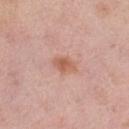This lesion was catalogued during total-body skin photography and was not selected for biopsy. The lesion is located on the left thigh. A close-up tile cropped from a whole-body skin photograph, about 15 mm across. A female subject aged 48–52.